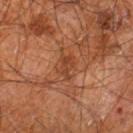  biopsy_status: not biopsied; imaged during a skin examination
  automated_metrics:
    shape_asymmetry: 0.4
  lighting: cross-polarized
  patient:
    sex: male
    age_approx: 60
  lesion_size:
    long_diameter_mm_approx: 3.5
  site: right leg
  image:
    source: total-body photography crop
    field_of_view_mm: 15